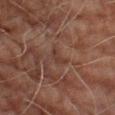| feature | finding |
|---|---|
| workup | imaged on a skin check; not biopsied |
| subject | male, aged 68–72 |
| illumination | cross-polarized |
| TBP lesion metrics | a within-lesion color-variation index near 0/10 and a peripheral color-asymmetry measure near 0 |
| anatomic site | the right lower leg |
| image | ~15 mm tile from a whole-body skin photo |
| diameter | ≈2.5 mm |